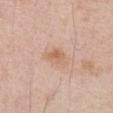Recorded during total-body skin imaging; not selected for excision or biopsy. A male subject, in their 70s. A roughly 15 mm field-of-view crop from a total-body skin photograph. The lesion is on the abdomen.